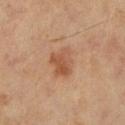Q: Was a biopsy performed?
A: catalogued during a skin exam; not biopsied
Q: Lesion location?
A: the right lower leg
Q: Who is the patient?
A: male, aged around 60
Q: Lesion size?
A: about 3.5 mm
Q: What lighting was used for the tile?
A: cross-polarized illumination
Q: What kind of image is this?
A: ~15 mm tile from a whole-body skin photo